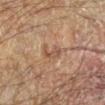biopsy_status: not biopsied; imaged during a skin examination
site: left lower leg
image:
  source: total-body photography crop
  field_of_view_mm: 15
patient:
  sex: male
  age_approx: 65
lighting: cross-polarized
lesion_size:
  long_diameter_mm_approx: 3.0
automated_metrics:
  border_irregularity_0_10: 4.0
  color_variation_0_10: 4.0
  peripheral_color_asymmetry: 1.5